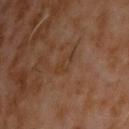* follow-up · no biopsy performed (imaged during a skin exam)
* image · ~15 mm crop, total-body skin-cancer survey
* lighting · cross-polarized illumination
* TBP lesion metrics · a footprint of about 3 mm², an outline eccentricity of about 0.85 (0 = round, 1 = elongated), and two-axis asymmetry of about 0.4; an average lesion color of about L≈34 a*≈17 b*≈28 (CIELAB), about 4 CIELAB-L* units darker than the surrounding skin, and a normalized border contrast of about 4.5; a border-irregularity rating of about 4/10, a within-lesion color-variation index near 1/10, and radial color variation of about 0
* subject · male, in their 60s
* anatomic site · the upper back
* lesion size · ~2.5 mm (longest diameter)